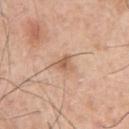– follow-up · catalogued during a skin exam; not biopsied
– subject · male, about 70 years old
– image source · total-body-photography crop, ~15 mm field of view
– body site · the left upper arm